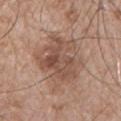This lesion was catalogued during total-body skin photography and was not selected for biopsy.
This is a white-light tile.
About 7 mm across.
A close-up tile cropped from a whole-body skin photograph, about 15 mm across.
From the chest.
The subject is a male in their mid-60s.